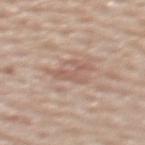{"biopsy_status": "not biopsied; imaged during a skin examination", "lesion_size": {"long_diameter_mm_approx": 4.0}, "patient": {"sex": "female", "age_approx": 60}, "automated_metrics": {"border_irregularity_0_10": 10.0, "color_variation_0_10": 0.5, "lesion_detection_confidence_0_100": 65}, "site": "back", "image": {"source": "total-body photography crop", "field_of_view_mm": 15}, "lighting": "white-light"}